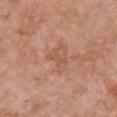| field | value |
|---|---|
| follow-up | imaged on a skin check; not biopsied |
| patient | female, about 60 years old |
| image source | ~15 mm tile from a whole-body skin photo |
| anatomic site | the chest |